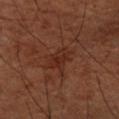follow-up — catalogued during a skin exam; not biopsied
image — 15 mm crop, total-body photography
subject — male, roughly 55 years of age
body site — the arm
lighting — cross-polarized illumination
lesion diameter — ≈4 mm
TBP lesion metrics — an area of roughly 6.5 mm², an eccentricity of roughly 0.65, and two-axis asymmetry of about 0.45; a lesion color around L≈29 a*≈23 b*≈28 in CIELAB, about 6 CIELAB-L* units darker than the surrounding skin, and a normalized border contrast of about 6; a classifier nevus-likeness of about 0/100 and a lesion-detection confidence of about 100/100